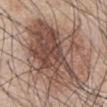Image and clinical context: This image is a 15 mm lesion crop taken from a total-body photograph. On the chest. Captured under white-light illumination. A male subject aged approximately 45. Measured at roughly 10 mm in maximum diameter.